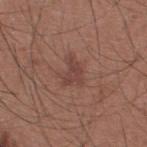The lesion is on the upper back. The total-body-photography lesion software estimated an average lesion color of about L≈42 a*≈21 b*≈24 (CIELAB), about 7 CIELAB-L* units darker than the surrounding skin, and a normalized lesion–skin contrast near 6. The tile uses white-light illumination. A male patient, approximately 35 years of age. A 15 mm crop from a total-body photograph taken for skin-cancer surveillance. The lesion's longest dimension is about 3.5 mm.